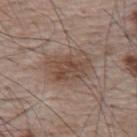Captured during whole-body skin photography for melanoma surveillance; the lesion was not biopsied.
Longest diameter approximately 5 mm.
The patient is a male in their mid-60s.
The total-body-photography lesion software estimated an average lesion color of about L≈48 a*≈16 b*≈25 (CIELAB), about 8 CIELAB-L* units darker than the surrounding skin, and a normalized lesion–skin contrast near 6.5. And it measured a border-irregularity rating of about 2.5/10 and a color-variation rating of about 4/10. It also reported a lesion-detection confidence of about 100/100.
A region of skin cropped from a whole-body photographic capture, roughly 15 mm wide.
The lesion is located on the upper back.
Captured under white-light illumination.